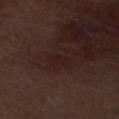Clinical impression:
Recorded during total-body skin imaging; not selected for excision or biopsy.
Acquisition and patient details:
This image is a 15 mm lesion crop taken from a total-body photograph. A male patient aged 68–72. Located on the left lower leg.